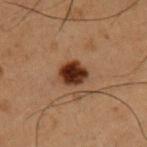notes: imaged on a skin check; not biopsied | image-analysis metrics: an area of roughly 7 mm², an eccentricity of roughly 0.55, and a shape-asymmetry score of about 0.2 (0 = symmetric); a border-irregularity index near 1.5/10 and internal color variation of about 4 on a 0–10 scale | anatomic site: the front of the torso | diameter: about 3.5 mm | tile lighting: cross-polarized illumination | imaging modality: total-body-photography crop, ~15 mm field of view | patient: male, aged approximately 50.